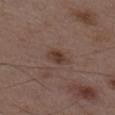<lesion>
<biopsy_status>not biopsied; imaged during a skin examination</biopsy_status>
<image>
  <source>total-body photography crop</source>
  <field_of_view_mm>15</field_of_view_mm>
</image>
<site>mid back</site>
<patient>
  <sex>male</sex>
  <age_approx>50</age_approx>
</patient>
</lesion>